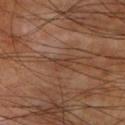The lesion was tiled from a total-body skin photograph and was not biopsied.
The lesion is on the left lower leg.
The tile uses cross-polarized illumination.
Cropped from a whole-body photographic skin survey; the tile spans about 15 mm.
Longest diameter approximately 2.5 mm.
A male subject, aged approximately 60.